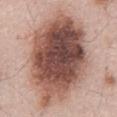notes: total-body-photography surveillance lesion; no biopsy
lighting: white-light
image-analysis metrics: a mean CIELAB color near L≈50 a*≈21 b*≈25, roughly 20 lightness units darker than nearby skin, and a normalized border contrast of about 13; a classifier nevus-likeness of about 45/100 and lesion-presence confidence of about 100/100
subject: male, aged approximately 55
diameter: about 13.5 mm
anatomic site: the front of the torso
imaging modality: ~15 mm crop, total-body skin-cancer survey